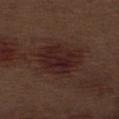workup: imaged on a skin check; not biopsied
subject: male, aged approximately 70
automated metrics: a footprint of about 19 mm², an eccentricity of roughly 0.6, and two-axis asymmetry of about 0.25; a mean CIELAB color near L≈23 a*≈19 b*≈18, about 7 CIELAB-L* units darker than the surrounding skin, and a normalized border contrast of about 8.5; border irregularity of about 2.5 on a 0–10 scale and internal color variation of about 4 on a 0–10 scale
imaging modality: ~15 mm tile from a whole-body skin photo
location: the left thigh